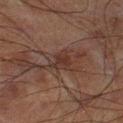Recorded during total-body skin imaging; not selected for excision or biopsy.
A close-up tile cropped from a whole-body skin photograph, about 15 mm across.
The total-body-photography lesion software estimated a border-irregularity index near 4.5/10. The software also gave a detector confidence of about 50 out of 100 that the crop contains a lesion.
From the leg.
A male subject aged around 70.
About 3 mm across.
The tile uses cross-polarized illumination.